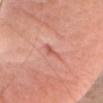workup — total-body-photography surveillance lesion; no biopsy
anatomic site — the head or neck
image source — ~15 mm tile from a whole-body skin photo
illumination — white-light illumination
diameter — ~3 mm (longest diameter)
patient — female, approximately 40 years of age
image-analysis metrics — a lesion area of about 2.5 mm², a shape eccentricity near 0.95, and a shape-asymmetry score of about 0.45 (0 = symmetric); a border-irregularity rating of about 5.5/10, a color-variation rating of about 0/10, and radial color variation of about 0; a nevus-likeness score of about 0/100 and a detector confidence of about 65 out of 100 that the crop contains a lesion